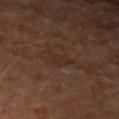Recorded during total-body skin imaging; not selected for excision or biopsy.
Located on the left forearm.
A male subject in their mid-50s.
Automated tile analysis of the lesion measured a lesion area of about 4 mm², a shape eccentricity near 0.9, and a symmetry-axis asymmetry near 0.5.
The lesion's longest dimension is about 3.5 mm.
A roughly 15 mm field-of-view crop from a total-body skin photograph.
This is a cross-polarized tile.